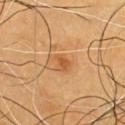biopsy status: imaged on a skin check; not biopsied
image: ~15 mm crop, total-body skin-cancer survey
illumination: cross-polarized illumination
location: the chest
subject: male, in their 60s
lesion diameter: about 2.5 mm